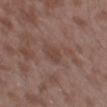Findings:
• biopsy status — total-body-photography surveillance lesion; no biopsy
• subject — male, aged 43 to 47
• diameter — about 3 mm
• tile lighting — white-light
• anatomic site — the left thigh
• acquisition — total-body-photography crop, ~15 mm field of view